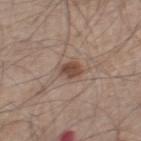biopsy status — catalogued during a skin exam; not biopsied | patient — male, roughly 60 years of age | acquisition — 15 mm crop, total-body photography | location — the right thigh.